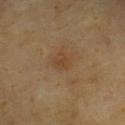| key | value |
|---|---|
| notes | total-body-photography surveillance lesion; no biopsy |
| tile lighting | cross-polarized illumination |
| acquisition | ~15 mm tile from a whole-body skin photo |
| location | the chest |
| size | about 1 mm |
| subject | male, in their mid-60s |